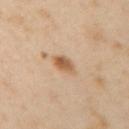Longest diameter approximately 3 mm.
A female patient roughly 40 years of age.
The lesion-visualizer software estimated border irregularity of about 2 on a 0–10 scale.
A close-up tile cropped from a whole-body skin photograph, about 15 mm across.
Located on the left arm.
The tile uses cross-polarized illumination.